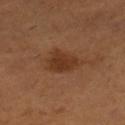Impression: Recorded during total-body skin imaging; not selected for excision or biopsy. Background: The lesion is on the right lower leg. Automated tile analysis of the lesion measured a mean CIELAB color near L≈35 a*≈21 b*≈32, a lesion–skin lightness drop of about 8, and a normalized border contrast of about 7.5. It also reported a nevus-likeness score of about 90/100 and a detector confidence of about 100 out of 100 that the crop contains a lesion. A female patient roughly 55 years of age. About 4 mm across. A 15 mm close-up tile from a total-body photography series done for melanoma screening. Captured under cross-polarized illumination.